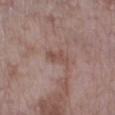Impression: This lesion was catalogued during total-body skin photography and was not selected for biopsy. Background: A female patient, aged 73–77. The lesion is on the left lower leg. This image is a 15 mm lesion crop taken from a total-body photograph.